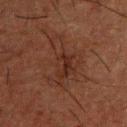– follow-up: imaged on a skin check; not biopsied
– anatomic site: the upper back
– illumination: cross-polarized illumination
– patient: male, aged around 50
– image-analysis metrics: a lesion color around L≈23 a*≈17 b*≈21 in CIELAB, a lesion–skin lightness drop of about 5, and a normalized border contrast of about 5.5; border irregularity of about 9 on a 0–10 scale, internal color variation of about 4 on a 0–10 scale, and peripheral color asymmetry of about 1; a classifier nevus-likeness of about 10/100 and a lesion-detection confidence of about 100/100
– imaging modality: ~15 mm tile from a whole-body skin photo
– lesion diameter: ~6.5 mm (longest diameter)